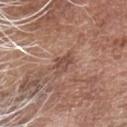<tbp_lesion>
<biopsy_status>not biopsied; imaged during a skin examination</biopsy_status>
<site>head or neck</site>
<automated_metrics>
  <cielab_L>48</cielab_L>
  <cielab_a>21</cielab_a>
  <cielab_b>26</cielab_b>
  <vs_skin_darker_L>9.0</vs_skin_darker_L>
  <vs_skin_contrast_norm>6.5</vs_skin_contrast_norm>
  <border_irregularity_0_10>3.0</border_irregularity_0_10>
  <color_variation_0_10>2.5</color_variation_0_10>
  <peripheral_color_asymmetry>1.0</peripheral_color_asymmetry>
  <nevus_likeness_0_100>0</nevus_likeness_0_100>
  <lesion_detection_confidence_0_100>100</lesion_detection_confidence_0_100>
</automated_metrics>
<image>
  <source>total-body photography crop</source>
  <field_of_view_mm>15</field_of_view_mm>
</image>
<patient>
  <sex>male</sex>
  <age_approx>75</age_approx>
</patient>
<lesion_size>
  <long_diameter_mm_approx>3.0</long_diameter_mm_approx>
</lesion_size>
<lighting>white-light</lighting>
</tbp_lesion>